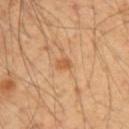patient:
  sex: male
  age_approx: 55
image:
  source: total-body photography crop
  field_of_view_mm: 15
site: upper back